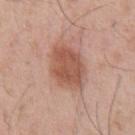follow-up: catalogued during a skin exam; not biopsied | acquisition: ~15 mm tile from a whole-body skin photo | patient: male, aged approximately 55 | site: the abdomen.